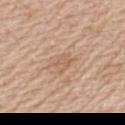The lesion was photographed on a routine skin check and not biopsied; there is no pathology result. On the upper back. A male subject, roughly 60 years of age. A roughly 15 mm field-of-view crop from a total-body skin photograph.